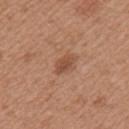Findings:
– notes: imaged on a skin check; not biopsied
– location: the left upper arm
– patient: female, approximately 35 years of age
– TBP lesion metrics: a border-irregularity rating of about 2.5/10, a within-lesion color-variation index near 1.5/10, and peripheral color asymmetry of about 0.5
– lighting: white-light
– acquisition: total-body-photography crop, ~15 mm field of view